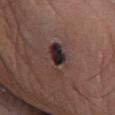Imaged during a routine full-body skin examination; the lesion was not biopsied and no histopathology is available. The lesion-visualizer software estimated a lesion color around L≈29 a*≈14 b*≈15 in CIELAB, a lesion–skin lightness drop of about 13, and a normalized border contrast of about 13. The software also gave a border-irregularity index near 5/10, a color-variation rating of about 9.5/10, and peripheral color asymmetry of about 3.5. And it measured an automated nevus-likeness rating near 0 out of 100 and a detector confidence of about 100 out of 100 that the crop contains a lesion. A close-up tile cropped from a whole-body skin photograph, about 15 mm across. Measured at roughly 4 mm in maximum diameter. A male subject, in their mid-60s. The lesion is on the leg.